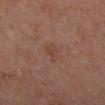Impression:
No biopsy was performed on this lesion — it was imaged during a full skin examination and was not determined to be concerning.
Image and clinical context:
The patient is a male approximately 65 years of age. On the left lower leg. Automated image analysis of the tile measured a lesion area of about 3.5 mm², an outline eccentricity of about 0.8 (0 = round, 1 = elongated), and two-axis asymmetry of about 0.3. Captured under cross-polarized illumination. A close-up tile cropped from a whole-body skin photograph, about 15 mm across.